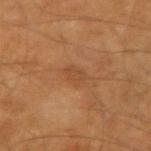image-analysis metrics: an eccentricity of roughly 0.75 and two-axis asymmetry of about 0.25; a border-irregularity rating of about 2.5/10 and a color-variation rating of about 1/10 | site: the left upper arm | lesion size: about 2.5 mm | acquisition: ~15 mm tile from a whole-body skin photo | patient: male, in their mid- to late 60s | lighting: cross-polarized illumination.